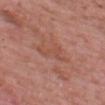{"patient": {"sex": "male", "age_approx": 65}, "image": {"source": "total-body photography crop", "field_of_view_mm": 15}, "site": "head or neck", "lighting": "white-light"}